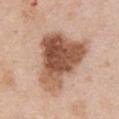<tbp_lesion>
<biopsy_status>not biopsied; imaged during a skin examination</biopsy_status>
<lighting>white-light</lighting>
<patient>
  <sex>female</sex>
  <age_approx>60</age_approx>
</patient>
<lesion_size>
  <long_diameter_mm_approx>8.0</long_diameter_mm_approx>
</lesion_size>
<site>chest</site>
<image>
  <source>total-body photography crop</source>
  <field_of_view_mm>15</field_of_view_mm>
</image>
</tbp_lesion>